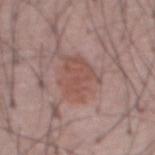This lesion was catalogued during total-body skin photography and was not selected for biopsy. The lesion is located on the abdomen. An algorithmic analysis of the crop reported a lesion–skin lightness drop of about 7 and a lesion-to-skin contrast of about 6 (normalized; higher = more distinct). The analysis additionally found border irregularity of about 5 on a 0–10 scale, a color-variation rating of about 2/10, and radial color variation of about 0.5. Captured under white-light illumination. A male patient roughly 55 years of age. This image is a 15 mm lesion crop taken from a total-body photograph. Longest diameter approximately 5 mm.